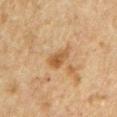biopsy status = catalogued during a skin exam; not biopsied
subject = male, about 50 years old
acquisition = total-body-photography crop, ~15 mm field of view
site = the left upper arm